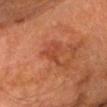workup = catalogued during a skin exam; not biopsied
automated metrics = an area of roughly 5 mm², a shape eccentricity near 0.65, and a symmetry-axis asymmetry near 0.55; an average lesion color of about L≈37 a*≈26 b*≈30 (CIELAB), a lesion–skin lightness drop of about 6, and a normalized lesion–skin contrast near 5.5; an automated nevus-likeness rating near 0 out of 100 and lesion-presence confidence of about 100/100
image = ~15 mm tile from a whole-body skin photo
subject = male, approximately 75 years of age
anatomic site = the chest
illumination = cross-polarized illumination